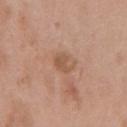{"biopsy_status": "not biopsied; imaged during a skin examination", "site": "chest", "automated_metrics": {"area_mm2_approx": 4.5, "eccentricity": 0.6, "lesion_detection_confidence_0_100": 100}, "patient": {"sex": "male", "age_approx": 65}, "image": {"source": "total-body photography crop", "field_of_view_mm": 15}, "lighting": "white-light"}